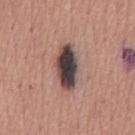Captured during whole-body skin photography for melanoma surveillance; the lesion was not biopsied. An algorithmic analysis of the crop reported an area of roughly 12 mm² and a shape eccentricity near 0.85. The software also gave roughly 20 lightness units darker than nearby skin and a lesion-to-skin contrast of about 15.5 (normalized; higher = more distinct). A male subject in their mid-40s. About 5.5 mm across. A 15 mm close-up tile from a total-body photography series done for melanoma screening. Captured under white-light illumination. From the chest.